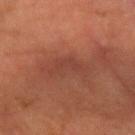* follow-up · catalogued during a skin exam; not biopsied
* image-analysis metrics · two-axis asymmetry of about 0.45; a classifier nevus-likeness of about 0/100 and a lesion-detection confidence of about 90/100
* illumination · cross-polarized illumination
* subject · male, about 55 years old
* lesion size · about 5 mm
* anatomic site · the right forearm
* imaging modality · total-body-photography crop, ~15 mm field of view